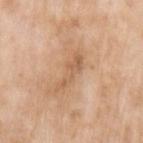The lesion is located on the left upper arm. Cropped from a whole-body photographic skin survey; the tile spans about 15 mm. A female patient, about 75 years old.